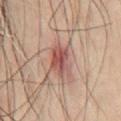The lesion was tiled from a total-body skin photograph and was not biopsied.